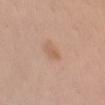Impression: Captured during whole-body skin photography for melanoma surveillance; the lesion was not biopsied. Background: The total-body-photography lesion software estimated a lesion area of about 2.5 mm², an eccentricity of roughly 0.85, and two-axis asymmetry of about 0.3. The analysis additionally found a lesion color around L≈60 a*≈20 b*≈31 in CIELAB. A female patient aged approximately 30. Approximately 2.5 mm at its widest. From the chest. A roughly 15 mm field-of-view crop from a total-body skin photograph.